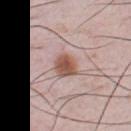Q: Is there a histopathology result?
A: imaged on a skin check; not biopsied
Q: Illumination type?
A: white-light illumination
Q: What did automated image analysis measure?
A: a border-irregularity rating of about 2/10 and a color-variation rating of about 3.5/10; a nevus-likeness score of about 100/100 and a lesion-detection confidence of about 100/100
Q: Where on the body is the lesion?
A: the chest
Q: What kind of image is this?
A: 15 mm crop, total-body photography
Q: Patient demographics?
A: male, aged approximately 35
Q: Lesion size?
A: ≈3 mm